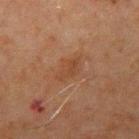No biopsy was performed on this lesion — it was imaged during a full skin examination and was not determined to be concerning. A male subject about 70 years old. Measured at roughly 3.5 mm in maximum diameter. Cropped from a total-body skin-imaging series; the visible field is about 15 mm. The tile uses cross-polarized illumination. Automated tile analysis of the lesion measured an area of roughly 5.5 mm² and a shape eccentricity near 0.8. And it measured a border-irregularity index near 3/10 and a color-variation rating of about 2/10. It also reported a classifier nevus-likeness of about 0/100 and a lesion-detection confidence of about 100/100. From the right upper arm.